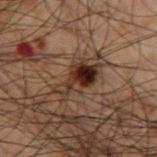The lesion was tiled from a total-body skin photograph and was not biopsied. The tile uses cross-polarized illumination. Cropped from a whole-body photographic skin survey; the tile spans about 15 mm. The subject is a male aged around 50. Automated image analysis of the tile measured a lesion color around L≈22 a*≈14 b*≈20 in CIELAB and a lesion–skin lightness drop of about 11. It also reported lesion-presence confidence of about 100/100. The lesion is located on the left thigh.